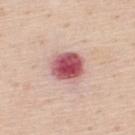Notes:
• notes · total-body-photography surveillance lesion; no biopsy
• image source · ~15 mm crop, total-body skin-cancer survey
• illumination · white-light illumination
• patient · male, aged 43–47
• automated metrics · a mean CIELAB color near L≈55 a*≈31 b*≈21, about 19 CIELAB-L* units darker than the surrounding skin, and a lesion-to-skin contrast of about 12 (normalized; higher = more distinct)
• site · the back